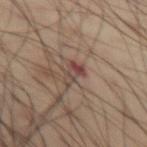From the abdomen. A male patient aged 53–57. This is a cross-polarized tile. This image is a 15 mm lesion crop taken from a total-body photograph. Approximately 3 mm at its widest.